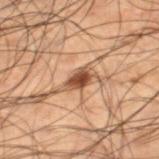Context: The lesion is on the right lower leg. An algorithmic analysis of the crop reported a lesion area of about 4.5 mm², an eccentricity of roughly 0.7, and a symmetry-axis asymmetry near 0.3. It also reported a lesion color around L≈37 a*≈18 b*≈26 in CIELAB, a lesion–skin lightness drop of about 13, and a normalized border contrast of about 11. It also reported an automated nevus-likeness rating near 95 out of 100. A 15 mm close-up tile from a total-body photography series done for melanoma screening. A male patient in their 50s. Imaged with cross-polarized lighting.